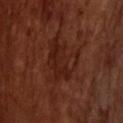Part of a total-body skin-imaging series; this lesion was reviewed on a skin check and was not flagged for biopsy.
A 15 mm close-up tile from a total-body photography series done for melanoma screening.
The lesion is on the head or neck.
A male subject about 70 years old.
This is a cross-polarized tile.